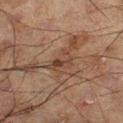This lesion was catalogued during total-body skin photography and was not selected for biopsy.
A male subject, aged 58–62.
The lesion is located on the left leg.
A roughly 15 mm field-of-view crop from a total-body skin photograph.